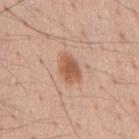Imaged during a routine full-body skin examination; the lesion was not biopsied and no histopathology is available.
The lesion-visualizer software estimated a color-variation rating of about 3/10 and radial color variation of about 1.
A male patient in their mid- to late 50s.
The tile uses white-light illumination.
The lesion is on the chest.
The lesion's longest dimension is about 3.5 mm.
A roughly 15 mm field-of-view crop from a total-body skin photograph.